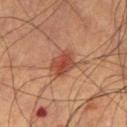* notes: catalogued during a skin exam; not biopsied
* patient: male, aged 68 to 72
* image: ~15 mm crop, total-body skin-cancer survey
* illumination: cross-polarized
* anatomic site: the left thigh
* lesion size: about 3.5 mm
* image-analysis metrics: a lesion area of about 8 mm², an eccentricity of roughly 0.6, and a symmetry-axis asymmetry near 0.15; a mean CIELAB color near L≈46 a*≈26 b*≈31 and about 11 CIELAB-L* units darker than the surrounding skin; a color-variation rating of about 4/10